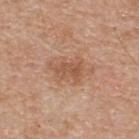This lesion was catalogued during total-body skin photography and was not selected for biopsy. On the back. An algorithmic analysis of the crop reported a footprint of about 12 mm², an outline eccentricity of about 0.75 (0 = round, 1 = elongated), and a symmetry-axis asymmetry near 0.25. The software also gave a border-irregularity index near 3.5/10 and radial color variation of about 1. The software also gave a classifier nevus-likeness of about 0/100 and lesion-presence confidence of about 100/100. A region of skin cropped from a whole-body photographic capture, roughly 15 mm wide. Captured under white-light illumination. The patient is a male about 55 years old.